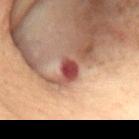Cropped from a whole-body photographic skin survey; the tile spans about 15 mm.
A female patient, roughly 80 years of age.
Located on the mid back.
Longest diameter approximately 3 mm.